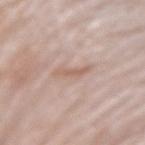notes = no biopsy performed (imaged during a skin exam) | acquisition = 15 mm crop, total-body photography | patient = male, in their mid-70s | lesion size = ≈3.5 mm | image-analysis metrics = a mean CIELAB color near L≈61 a*≈18 b*≈26, about 7 CIELAB-L* units darker than the surrounding skin, and a normalized lesion–skin contrast near 5.5; border irregularity of about 4.5 on a 0–10 scale | anatomic site = the arm.